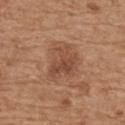Recorded during total-body skin imaging; not selected for excision or biopsy. A 15 mm close-up extracted from a 3D total-body photography capture. The lesion is located on the back. A male patient aged 68 to 72.